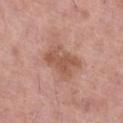| feature | finding |
|---|---|
| illumination | white-light |
| patient | female, aged around 60 |
| automated lesion analysis | a footprint of about 11 mm², a shape eccentricity near 0.7, and a symmetry-axis asymmetry near 0.3; a lesion color around L≈54 a*≈23 b*≈29 in CIELAB and a lesion–skin lightness drop of about 9 |
| diameter | ≈4.5 mm |
| location | the leg |
| imaging modality | 15 mm crop, total-body photography |